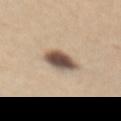| field | value |
|---|---|
| workup | imaged on a skin check; not biopsied |
| automated metrics | an eccentricity of roughly 0.55 and two-axis asymmetry of about 0.15; a mean CIELAB color near L≈52 a*≈14 b*≈25 and a lesion-to-skin contrast of about 13 (normalized; higher = more distinct); border irregularity of about 1.5 on a 0–10 scale, a within-lesion color-variation index near 7/10, and radial color variation of about 2; an automated nevus-likeness rating near 90 out of 100 and a lesion-detection confidence of about 100/100 |
| lighting | white-light |
| patient | female, in their 60s |
| imaging modality | ~15 mm tile from a whole-body skin photo |
| anatomic site | the abdomen |